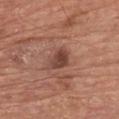Recorded during total-body skin imaging; not selected for excision or biopsy.
The total-body-photography lesion software estimated a mean CIELAB color near L≈43 a*≈22 b*≈26 and a normalized border contrast of about 8.
On the chest.
A male subject, roughly 80 years of age.
A 15 mm close-up extracted from a 3D total-body photography capture.
The tile uses white-light illumination.